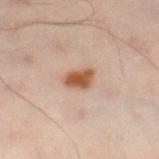biopsy status: no biopsy performed (imaged during a skin exam).